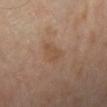The lesion was photographed on a routine skin check and not biopsied; there is no pathology result.
The lesion is located on the left lower leg.
The tile uses cross-polarized illumination.
The recorded lesion diameter is about 3 mm.
A 15 mm close-up extracted from a 3D total-body photography capture.
A female subject approximately 70 years of age.
The lesion-visualizer software estimated an area of roughly 3.5 mm², an eccentricity of roughly 0.75, and a shape-asymmetry score of about 0.45 (0 = symmetric). The software also gave a mean CIELAB color near L≈50 a*≈18 b*≈31 and a normalized border contrast of about 5.